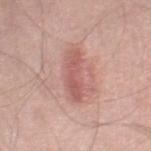| field | value |
|---|---|
| biopsy status | no biopsy performed (imaged during a skin exam) |
| size | ≈5.5 mm |
| image source | ~15 mm tile from a whole-body skin photo |
| anatomic site | the right thigh |
| subject | male, roughly 55 years of age |
| TBP lesion metrics | a lesion area of about 10 mm², an outline eccentricity of about 0.9 (0 = round, 1 = elongated), and a shape-asymmetry score of about 0.3 (0 = symmetric); an average lesion color of about L≈58 a*≈25 b*≈25 (CIELAB), a lesion–skin lightness drop of about 10, and a lesion-to-skin contrast of about 6.5 (normalized; higher = more distinct) |
| tile lighting | white-light illumination |